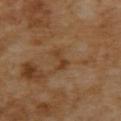Impression: The lesion was tiled from a total-body skin photograph and was not biopsied. Acquisition and patient details: This is a cross-polarized tile. A 15 mm close-up extracted from a 3D total-body photography capture. A female subject, aged 53 to 57. Measured at roughly 3 mm in maximum diameter. The lesion-visualizer software estimated an average lesion color of about L≈40 a*≈20 b*≈34 (CIELAB), about 8 CIELAB-L* units darker than the surrounding skin, and a normalized lesion–skin contrast near 7. The software also gave border irregularity of about 5.5 on a 0–10 scale and radial color variation of about 0. From the mid back.